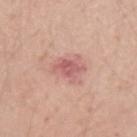Q: Lesion size?
A: ~3 mm (longest diameter)
Q: How was the tile lit?
A: white-light illumination
Q: What is the anatomic site?
A: the arm
Q: What is the imaging modality?
A: ~15 mm crop, total-body skin-cancer survey
Q: Who is the patient?
A: male, approximately 30 years of age